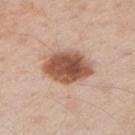Findings:
- follow-up — catalogued during a skin exam; not biopsied
- body site — the left upper arm
- acquisition — ~15 mm crop, total-body skin-cancer survey
- subject — male, aged around 55
- lesion diameter — ≈5.5 mm
- lighting — white-light illumination